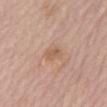{"biopsy_status": "not biopsied; imaged during a skin examination"}